Located on the left lower leg. A close-up tile cropped from a whole-body skin photograph, about 15 mm across. A female subject aged around 70 — 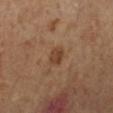The tile uses cross-polarized illumination. The total-body-photography lesion software estimated a shape eccentricity near 0.5 and two-axis asymmetry of about 0.15. And it measured a lesion color around L≈42 a*≈20 b*≈32 in CIELAB, a lesion–skin lightness drop of about 9, and a normalized lesion–skin contrast near 7. The recorded lesion diameter is about 2 mm. On biopsy, histopathology showed a malignancy: superficial basal cell carcinoma.